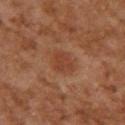Impression:
No biopsy was performed on this lesion — it was imaged during a full skin examination and was not determined to be concerning.
Background:
A female subject approximately 60 years of age. From the upper back. A lesion tile, about 15 mm wide, cut from a 3D total-body photograph.